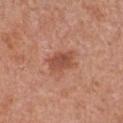This lesion was catalogued during total-body skin photography and was not selected for biopsy.
Located on the chest.
A female subject, aged 48 to 52.
A lesion tile, about 15 mm wide, cut from a 3D total-body photograph.
The total-body-photography lesion software estimated border irregularity of about 2.5 on a 0–10 scale, a within-lesion color-variation index near 2.5/10, and radial color variation of about 0.5. The analysis additionally found an automated nevus-likeness rating near 80 out of 100 and lesion-presence confidence of about 100/100.
The tile uses white-light illumination.